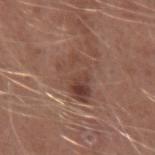This image is a 15 mm lesion crop taken from a total-body photograph. The recorded lesion diameter is about 5 mm. The tile uses white-light illumination. A male subject, aged 23 to 27. From the right forearm. The lesion-visualizer software estimated a footprint of about 12 mm², an eccentricity of roughly 0.75, and a shape-asymmetry score of about 0.3 (0 = symmetric). It also reported a lesion color around L≈43 a*≈20 b*≈25 in CIELAB, roughly 9 lightness units darker than nearby skin, and a lesion-to-skin contrast of about 7 (normalized; higher = more distinct). And it measured a classifier nevus-likeness of about 10/100 and a detector confidence of about 100 out of 100 that the crop contains a lesion.